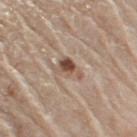Findings:
• follow-up — imaged on a skin check; not biopsied
• body site — the arm
• subject — male, about 75 years old
• size — ~3.5 mm (longest diameter)
• tile lighting — white-light illumination
• image — 15 mm crop, total-body photography